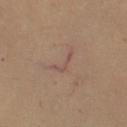- patient: female, aged approximately 40
- automated lesion analysis: an outline eccentricity of about 0.9 (0 = round, 1 = elongated) and a shape-asymmetry score of about 0.8 (0 = symmetric); a mean CIELAB color near L≈51 a*≈18 b*≈24, about 6 CIELAB-L* units darker than the surrounding skin, and a normalized border contrast of about 5
- anatomic site: the right upper arm
- size: about 4 mm
- imaging modality: 15 mm crop, total-body photography
- lighting: cross-polarized illumination